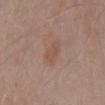Findings:
• subject: male, in their mid- to late 50s
• tile lighting: white-light
• image: 15 mm crop, total-body photography
• body site: the mid back
• lesion size: ~3.5 mm (longest diameter)